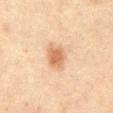automated metrics: an outline eccentricity of about 0.6 (0 = round, 1 = elongated) and a shape-asymmetry score of about 0.15 (0 = symmetric); border irregularity of about 1.5 on a 0–10 scale and peripheral color asymmetry of about 1
size: ~3 mm (longest diameter)
tile lighting: cross-polarized illumination
patient: male, about 50 years old
body site: the abdomen
imaging modality: ~15 mm crop, total-body skin-cancer survey